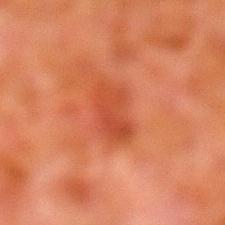Findings:
– workup — total-body-photography surveillance lesion; no biopsy
– subject — male, aged 78 to 82
– image — total-body-photography crop, ~15 mm field of view
– illumination — cross-polarized
– site — the left lower leg